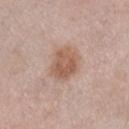This lesion was catalogued during total-body skin photography and was not selected for biopsy.
Approximately 4.5 mm at its widest.
Imaged with white-light lighting.
From the right lower leg.
The subject is a female aged around 65.
A region of skin cropped from a whole-body photographic capture, roughly 15 mm wide.